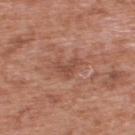Case summary:
* notes · catalogued during a skin exam; not biopsied
* anatomic site · the upper back
* patient · male, in their 70s
* imaging modality · total-body-photography crop, ~15 mm field of view
* lesion diameter · about 3 mm
* automated metrics · a footprint of about 4 mm², an outline eccentricity of about 0.75 (0 = round, 1 = elongated), and two-axis asymmetry of about 0.65; a mean CIELAB color near L≈48 a*≈23 b*≈29, a lesion–skin lightness drop of about 8, and a normalized border contrast of about 5.5; a classifier nevus-likeness of about 0/100 and a lesion-detection confidence of about 100/100
* tile lighting · white-light